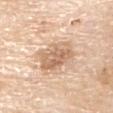Clinical impression: Recorded during total-body skin imaging; not selected for excision or biopsy. Context: This is a white-light tile. The lesion is on the left upper arm. A male subject, approximately 80 years of age. Longest diameter approximately 5 mm. A 15 mm close-up tile from a total-body photography series done for melanoma screening.